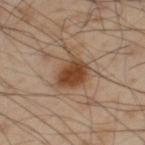| key | value |
|---|---|
| notes | imaged on a skin check; not biopsied |
| size | ~4.5 mm (longest diameter) |
| anatomic site | the left thigh |
| acquisition | total-body-photography crop, ~15 mm field of view |
| automated metrics | an average lesion color of about L≈44 a*≈19 b*≈31 (CIELAB) and a normalized border contrast of about 10 |
| subject | male, in their mid-50s |
| tile lighting | cross-polarized |